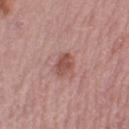Assessment:
Captured during whole-body skin photography for melanoma surveillance; the lesion was not biopsied.
Acquisition and patient details:
A female subject aged 48–52. The lesion is on the left thigh. A 15 mm crop from a total-body photograph taken for skin-cancer surveillance.